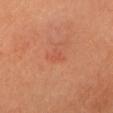Recorded during total-body skin imaging; not selected for excision or biopsy. The lesion is on the head or neck. A female patient. Cropped from a total-body skin-imaging series; the visible field is about 15 mm. The tile uses cross-polarized illumination. Approximately 1.5 mm at its widest. Automated image analysis of the tile measured a lesion color around L≈53 a*≈31 b*≈34 in CIELAB, a lesion–skin lightness drop of about 5, and a normalized lesion–skin contrast near 3.5. And it measured a border-irregularity rating of about 3/10, internal color variation of about 0 on a 0–10 scale, and radial color variation of about 0. The analysis additionally found a nevus-likeness score of about 0/100 and a detector confidence of about 100 out of 100 that the crop contains a lesion.